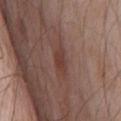Imaged during a routine full-body skin examination; the lesion was not biopsied and no histopathology is available. The total-body-photography lesion software estimated a border-irregularity index near 3/10, a within-lesion color-variation index near 2/10, and a peripheral color-asymmetry measure near 0.5. It also reported an automated nevus-likeness rating near 5 out of 100 and lesion-presence confidence of about 100/100. Imaged with white-light lighting. The lesion's longest dimension is about 3.5 mm. From the chest. A lesion tile, about 15 mm wide, cut from a 3D total-body photograph. A male patient about 65 years old.